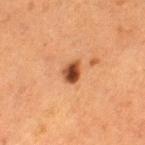Imaged during a routine full-body skin examination; the lesion was not biopsied and no histopathology is available.
A 15 mm close-up extracted from a 3D total-body photography capture.
The tile uses cross-polarized illumination.
The patient is a female approximately 50 years of age.
The lesion is on the left thigh.